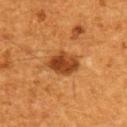Notes:
- follow-up · imaged on a skin check; not biopsied
- acquisition · ~15 mm crop, total-body skin-cancer survey
- patient · male, aged around 60
- tile lighting · cross-polarized
- lesion diameter · ≈4 mm
- anatomic site · the upper back